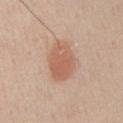biopsy status: imaged on a skin check; not biopsied | lesion size: ~5 mm (longest diameter) | patient: male, aged 28–32 | imaging modality: 15 mm crop, total-body photography | TBP lesion metrics: a shape eccentricity near 0.75 and two-axis asymmetry of about 0.15; a lesion-to-skin contrast of about 6.5 (normalized; higher = more distinct) | illumination: white-light | location: the chest.